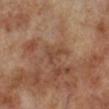workup: imaged on a skin check; not biopsied
body site: the left lower leg
tile lighting: cross-polarized
size: about 3.5 mm
imaging modality: total-body-photography crop, ~15 mm field of view
patient: male, approximately 70 years of age